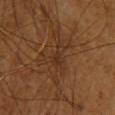This lesion was catalogued during total-body skin photography and was not selected for biopsy. This image is a 15 mm lesion crop taken from a total-body photograph. The lesion is located on the upper back. Captured under cross-polarized illumination. Automated image analysis of the tile measured an automated nevus-likeness rating near 0 out of 100 and a detector confidence of about 65 out of 100 that the crop contains a lesion. The patient is a female aged approximately 55.